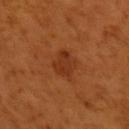| key | value |
|---|---|
| follow-up | catalogued during a skin exam; not biopsied |
| acquisition | ~15 mm crop, total-body skin-cancer survey |
| tile lighting | cross-polarized illumination |
| TBP lesion metrics | border irregularity of about 3 on a 0–10 scale, a within-lesion color-variation index near 2/10, and peripheral color asymmetry of about 0.5 |
| patient | male, aged approximately 50 |
| location | the left upper arm |
| lesion diameter | ~4 mm (longest diameter) |